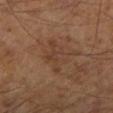workup: no biopsy performed (imaged during a skin exam)
diameter: ~5 mm (longest diameter)
imaging modality: 15 mm crop, total-body photography
automated lesion analysis: a border-irregularity rating of about 4.5/10, internal color variation of about 4 on a 0–10 scale, and peripheral color asymmetry of about 1; a classifier nevus-likeness of about 0/100 and a detector confidence of about 100 out of 100 that the crop contains a lesion
tile lighting: cross-polarized
patient: male, aged around 65